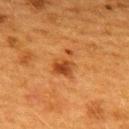A female subject, roughly 40 years of age. About 3.5 mm across. Located on the upper back. This is a cross-polarized tile. A lesion tile, about 15 mm wide, cut from a 3D total-body photograph.